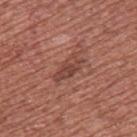workup: total-body-photography surveillance lesion; no biopsy
patient: male, in their mid- to late 40s
automated metrics: a shape eccentricity near 0.9 and a shape-asymmetry score of about 0.3 (0 = symmetric); a border-irregularity rating of about 3.5/10, internal color variation of about 3.5 on a 0–10 scale, and a peripheral color-asymmetry measure near 1.5; a lesion-detection confidence of about 95/100
site: the right upper arm
acquisition: 15 mm crop, total-body photography
lighting: white-light illumination